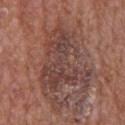Findings:
- lighting — white-light illumination
- image-analysis metrics — a lesion area of about 31 mm²; a mean CIELAB color near L≈42 a*≈20 b*≈22, roughly 8 lightness units darker than nearby skin, and a normalized border contrast of about 7; a border-irregularity rating of about 9/10, a within-lesion color-variation index near 4.5/10, and radial color variation of about 1.5
- image — ~15 mm tile from a whole-body skin photo
- patient — male, about 65 years old
- diameter — ~8.5 mm (longest diameter)
- location — the front of the torso